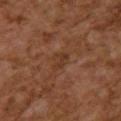Clinical impression:
Captured during whole-body skin photography for melanoma surveillance; the lesion was not biopsied.
Clinical summary:
From the upper back. A female patient roughly 60 years of age. This is a cross-polarized tile. A 15 mm close-up tile from a total-body photography series done for melanoma screening. The lesion's longest dimension is about 2.5 mm.